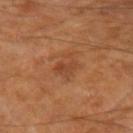| key | value |
|---|---|
| notes | total-body-photography surveillance lesion; no biopsy |
| location | the right forearm |
| acquisition | ~15 mm tile from a whole-body skin photo |
| automated metrics | a lesion area of about 5.5 mm², an eccentricity of roughly 0.55, and a shape-asymmetry score of about 0.4 (0 = symmetric); a lesion color around L≈43 a*≈24 b*≈34 in CIELAB, about 6 CIELAB-L* units darker than the surrounding skin, and a normalized lesion–skin contrast near 5; a border-irregularity index near 5.5/10, a color-variation rating of about 3/10, and a peripheral color-asymmetry measure near 1; a detector confidence of about 100 out of 100 that the crop contains a lesion |
| diameter | about 3 mm |
| illumination | cross-polarized illumination |
| subject | male, aged around 85 or older |